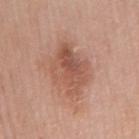Part of a total-body skin-imaging series; this lesion was reviewed on a skin check and was not flagged for biopsy. A 15 mm close-up tile from a total-body photography series done for melanoma screening. Automated tile analysis of the lesion measured a mean CIELAB color near L≈54 a*≈22 b*≈29, roughly 10 lightness units darker than nearby skin, and a normalized border contrast of about 7. It also reported an automated nevus-likeness rating near 15 out of 100 and lesion-presence confidence of about 100/100. The patient is a male aged 78–82. From the mid back.